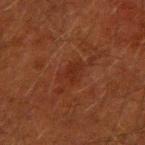Notes:
* anatomic site — the right upper arm
* image — total-body-photography crop, ~15 mm field of view
* subject — male, aged around 50
* automated metrics — a lesion color around L≈22 a*≈21 b*≈25 in CIELAB; a border-irregularity rating of about 4.5/10, a within-lesion color-variation index near 1.5/10, and peripheral color asymmetry of about 0.5; a classifier nevus-likeness of about 0/100 and a detector confidence of about 100 out of 100 that the crop contains a lesion